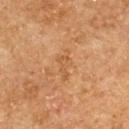Acquisition and patient details:
A female patient, aged around 60. This is a cross-polarized tile. On the upper back. Cropped from a total-body skin-imaging series; the visible field is about 15 mm. Automated image analysis of the tile measured an area of roughly 4.5 mm². The analysis additionally found an average lesion color of about L≈53 a*≈21 b*≈38 (CIELAB), roughly 6 lightness units darker than nearby skin, and a normalized lesion–skin contrast near 4.5. The analysis additionally found a border-irregularity rating of about 6/10, a color-variation rating of about 1.5/10, and peripheral color asymmetry of about 0.5.